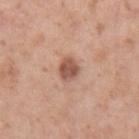Captured under white-light illumination.
Automated tile analysis of the lesion measured a within-lesion color-variation index near 2.5/10 and radial color variation of about 1. It also reported a classifier nevus-likeness of about 75/100 and a lesion-detection confidence of about 100/100.
From the right forearm.
Approximately 2.5 mm at its widest.
A female patient, in their 40s.
A 15 mm crop from a total-body photograph taken for skin-cancer surveillance.